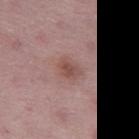| feature | finding |
|---|---|
| anatomic site | the left thigh |
| patient | female, aged 48–52 |
| image-analysis metrics | an outline eccentricity of about 0.65 (0 = round, 1 = elongated) and a symmetry-axis asymmetry near 0.2; a mean CIELAB color near L≈50 a*≈21 b*≈23, a lesion–skin lightness drop of about 8, and a normalized border contrast of about 6.5 |
| imaging modality | total-body-photography crop, ~15 mm field of view |
| lesion diameter | ~3 mm (longest diameter) |